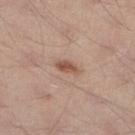{"biopsy_status": "not biopsied; imaged during a skin examination", "patient": {"sex": "male", "age_approx": 35}, "site": "right lower leg", "lighting": "white-light", "lesion_size": {"long_diameter_mm_approx": 3.0}, "image": {"source": "total-body photography crop", "field_of_view_mm": 15}, "automated_metrics": {"area_mm2_approx": 3.5, "shape_asymmetry": 0.35}}